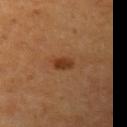Notes:
- biopsy status · no biopsy performed (imaged during a skin exam)
- patient · female, aged 53 to 57
- site · the left upper arm
- lighting · cross-polarized
- lesion size · ~3 mm (longest diameter)
- image source · ~15 mm crop, total-body skin-cancer survey
- image-analysis metrics · an area of roughly 3.5 mm², a shape eccentricity near 0.85, and two-axis asymmetry of about 0.2; an average lesion color of about L≈34 a*≈22 b*≈33 (CIELAB) and a lesion–skin lightness drop of about 10; an automated nevus-likeness rating near 100 out of 100